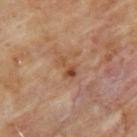Assessment: Recorded during total-body skin imaging; not selected for excision or biopsy. Context: The patient is a male aged around 65. Cropped from a whole-body photographic skin survey; the tile spans about 15 mm. This is a cross-polarized tile. The lesion is on the left upper arm. The lesion's longest dimension is about 3 mm.